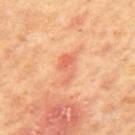<record>
<lighting>cross-polarized</lighting>
<image>
  <source>total-body photography crop</source>
  <field_of_view_mm>15</field_of_view_mm>
</image>
<lesion_size>
  <long_diameter_mm_approx>4.0</long_diameter_mm_approx>
</lesion_size>
<patient>
  <sex>male</sex>
  <age_approx>65</age_approx>
</patient>
<site>mid back</site>
</record>